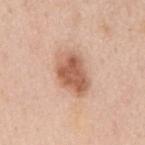Notes:
– biopsy status · total-body-photography surveillance lesion; no biopsy
– illumination · white-light illumination
– size · ~5.5 mm (longest diameter)
– body site · the mid back
– image source · ~15 mm tile from a whole-body skin photo
– subject · male, roughly 50 years of age